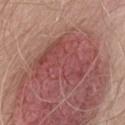On the lower back. A male subject, in their 60s. A region of skin cropped from a whole-body photographic capture, roughly 15 mm wide. Imaged with white-light lighting.